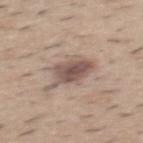Impression: The lesion was tiled from a total-body skin photograph and was not biopsied. Clinical summary: Longest diameter approximately 5.5 mm. Captured under white-light illumination. Automated image analysis of the tile measured an area of roughly 11 mm² and an outline eccentricity of about 0.75 (0 = round, 1 = elongated). The analysis additionally found a mean CIELAB color near L≈53 a*≈16 b*≈22, roughly 13 lightness units darker than nearby skin, and a lesion-to-skin contrast of about 9 (normalized; higher = more distinct). The analysis additionally found a nevus-likeness score of about 75/100 and a detector confidence of about 100 out of 100 that the crop contains a lesion. The subject is a male aged 38–42. A region of skin cropped from a whole-body photographic capture, roughly 15 mm wide. The lesion is on the mid back.